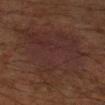Q: Was a biopsy performed?
A: total-body-photography surveillance lesion; no biopsy
Q: Where on the body is the lesion?
A: the right upper arm
Q: Automated lesion metrics?
A: an eccentricity of roughly 0.75 and two-axis asymmetry of about 0.35; a nevus-likeness score of about 5/100 and a detector confidence of about 95 out of 100 that the crop contains a lesion
Q: Illumination type?
A: cross-polarized illumination
Q: How was this image acquired?
A: ~15 mm crop, total-body skin-cancer survey
Q: Who is the patient?
A: male, about 60 years old